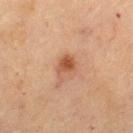Assessment: Part of a total-body skin-imaging series; this lesion was reviewed on a skin check and was not flagged for biopsy. Image and clinical context: Longest diameter approximately 3 mm. A female patient, aged approximately 70. The lesion is located on the left thigh. The tile uses cross-polarized illumination. A 15 mm close-up tile from a total-body photography series done for melanoma screening.